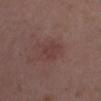Imaged during a routine full-body skin examination; the lesion was not biopsied and no histopathology is available.
A 15 mm close-up extracted from a 3D total-body photography capture.
A female patient aged 33 to 37.
The lesion is located on the right lower leg.